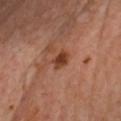Clinical impression:
The lesion was tiled from a total-body skin photograph and was not biopsied.